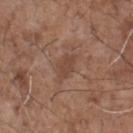Imaged during a routine full-body skin examination; the lesion was not biopsied and no histopathology is available. The lesion-visualizer software estimated a border-irregularity index near 2.5/10, a within-lesion color-variation index near 2/10, and a peripheral color-asymmetry measure near 1. The analysis additionally found an automated nevus-likeness rating near 5 out of 100 and a detector confidence of about 100 out of 100 that the crop contains a lesion. A close-up tile cropped from a whole-body skin photograph, about 15 mm across. On the chest. This is a white-light tile. A male patient, aged 68 to 72.